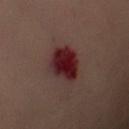Assessment: No biopsy was performed on this lesion — it was imaged during a full skin examination and was not determined to be concerning. Background: Imaged with cross-polarized lighting. About 4.5 mm across. A female subject in their 60s. Cropped from a whole-body photographic skin survey; the tile spans about 15 mm. The lesion is on the chest.